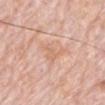Assessment: No biopsy was performed on this lesion — it was imaged during a full skin examination and was not determined to be concerning. Image and clinical context: A male patient, roughly 80 years of age. On the mid back. Measured at roughly 3.5 mm in maximum diameter. Imaged with white-light lighting. A lesion tile, about 15 mm wide, cut from a 3D total-body photograph. The total-body-photography lesion software estimated an area of roughly 5 mm² and a shape-asymmetry score of about 0.55 (0 = symmetric). The software also gave a border-irregularity rating of about 6.5/10, a within-lesion color-variation index near 1/10, and peripheral color asymmetry of about 0.5. It also reported a classifier nevus-likeness of about 0/100.